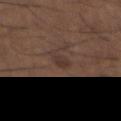The lesion was tiled from a total-body skin photograph and was not biopsied. A roughly 15 mm field-of-view crop from a total-body skin photograph. Located on the chest. The total-body-photography lesion software estimated a mean CIELAB color near L≈35 a*≈16 b*≈21 and roughly 6 lightness units darker than nearby skin. Longest diameter approximately 3 mm. Imaged with white-light lighting. The subject is a male in their 50s.